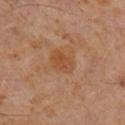Clinical impression:
Captured during whole-body skin photography for melanoma surveillance; the lesion was not biopsied.
Context:
From the right lower leg. A 15 mm close-up tile from a total-body photography series done for melanoma screening. The subject is a male aged approximately 60.